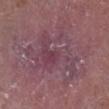No biopsy was performed on this lesion — it was imaged during a full skin examination and was not determined to be concerning. A male patient, aged approximately 75. A 15 mm crop from a total-body photograph taken for skin-cancer surveillance. The lesion's longest dimension is about 8.5 mm. The lesion is on the right lower leg. Automated image analysis of the tile measured a lesion area of about 31 mm² and a shape-asymmetry score of about 0.45 (0 = symmetric). And it measured a lesion–skin lightness drop of about 6 and a normalized lesion–skin contrast near 5.5. The software also gave border irregularity of about 8 on a 0–10 scale and a within-lesion color-variation index near 5.5/10. The software also gave a nevus-likeness score of about 0/100 and lesion-presence confidence of about 20/100.